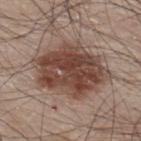notes: total-body-photography surveillance lesion; no biopsy | image source: 15 mm crop, total-body photography | subject: male, aged 43 to 47 | tile lighting: white-light | diameter: ≈8.5 mm | site: the upper back.